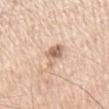follow-up=catalogued during a skin exam; not biopsied | subject=male, in their mid- to late 60s | automated lesion analysis=a lesion color around L≈65 a*≈17 b*≈30 in CIELAB, roughly 13 lightness units darker than nearby skin, and a normalized lesion–skin contrast near 7.5; internal color variation of about 4.5 on a 0–10 scale and a peripheral color-asymmetry measure near 1.5; a nevus-likeness score of about 30/100 and a lesion-detection confidence of about 100/100 | size=about 3.5 mm | illumination=white-light illumination | image=15 mm crop, total-body photography | location=the right upper arm.